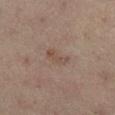Assessment:
Recorded during total-body skin imaging; not selected for excision or biopsy.
Context:
The subject is a male in their mid- to late 50s. Captured under cross-polarized illumination. A 15 mm close-up tile from a total-body photography series done for melanoma screening. From the right lower leg.